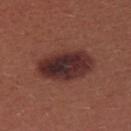  image:
    source: total-body photography crop
    field_of_view_mm: 15
  lesion_size:
    long_diameter_mm_approx: 6.5
  patient:
    sex: female
    age_approx: 25
  site: upper back
  lighting: white-light
  diagnosis:
    histopathology: atypical intraepithelial melanocytic proliferation
    malignancy: indeterminate
    taxonomic_path:
      - Indeterminate
      - Indeterminate melanocytic proliferations
      - Atypical intraepithelial melanocytic proliferation